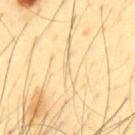Imaged during a routine full-body skin examination; the lesion was not biopsied and no histopathology is available.
On the mid back.
A male subject, aged approximately 45.
The lesion-visualizer software estimated an area of roughly 1 mm², an outline eccentricity of about 0.85 (0 = round, 1 = elongated), and two-axis asymmetry of about 0.4. And it measured an average lesion color of about L≈77 a*≈11 b*≈39 (CIELAB), about 5 CIELAB-L* units darker than the surrounding skin, and a normalized border contrast of about 3. The software also gave border irregularity of about 3.5 on a 0–10 scale and internal color variation of about 0 on a 0–10 scale.
A 15 mm close-up tile from a total-body photography series done for melanoma screening.
Longest diameter approximately 1.5 mm.